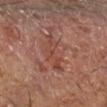No biopsy was performed on this lesion — it was imaged during a full skin examination and was not determined to be concerning.